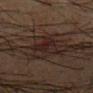follow-up = catalogued during a skin exam; not biopsied | anatomic site = the mid back | acquisition = ~15 mm crop, total-body skin-cancer survey | subject = male, aged approximately 65 | lesion size = ≈4.5 mm | tile lighting = cross-polarized illumination.